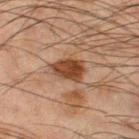* workup · no biopsy performed (imaged during a skin exam)
* site · the left thigh
* imaging modality · ~15 mm crop, total-body skin-cancer survey
* illumination · cross-polarized
* image-analysis metrics · an area of roughly 7.5 mm², an eccentricity of roughly 0.75, and two-axis asymmetry of about 0.25; a nevus-likeness score of about 90/100 and lesion-presence confidence of about 100/100
* diameter · ~4 mm (longest diameter)
* patient · male, about 65 years old